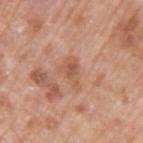Image and clinical context:
A male subject aged approximately 70. A region of skin cropped from a whole-body photographic capture, roughly 15 mm wide. On the left upper arm.